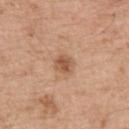The lesion is on the upper back.
Cropped from a whole-body photographic skin survey; the tile spans about 15 mm.
Measured at roughly 3 mm in maximum diameter.
The total-body-photography lesion software estimated an average lesion color of about L≈55 a*≈21 b*≈33 (CIELAB), a lesion–skin lightness drop of about 11, and a lesion-to-skin contrast of about 7.5 (normalized; higher = more distinct). The software also gave an automated nevus-likeness rating near 75 out of 100 and a lesion-detection confidence of about 100/100.
A male patient aged 53 to 57.